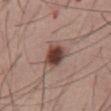Clinical impression: This lesion was catalogued during total-body skin photography and was not selected for biopsy. Context: Captured under white-light illumination. A male subject in their mid-50s. The lesion is on the abdomen. Approximately 3.5 mm at its widest. A 15 mm crop from a total-body photograph taken for skin-cancer surveillance.